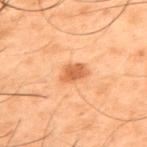The lesion was tiled from a total-body skin photograph and was not biopsied. This is a cross-polarized tile. The subject is a male roughly 50 years of age. A close-up tile cropped from a whole-body skin photograph, about 15 mm across. Located on the back. About 3.5 mm across.